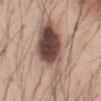This is a white-light tile. Automated tile analysis of the lesion measured a shape-asymmetry score of about 0.35 (0 = symmetric). It also reported an average lesion color of about L≈50 a*≈17 b*≈23 (CIELAB), roughly 17 lightness units darker than nearby skin, and a lesion-to-skin contrast of about 11.5 (normalized; higher = more distinct). And it measured border irregularity of about 5.5 on a 0–10 scale, a within-lesion color-variation index near 10/10, and a peripheral color-asymmetry measure near 3.5. The analysis additionally found a nevus-likeness score of about 100/100 and a detector confidence of about 100 out of 100 that the crop contains a lesion. The recorded lesion diameter is about 10.5 mm. This image is a 15 mm lesion crop taken from a total-body photograph. The lesion is on the abdomen. A male patient, in their mid-40s.